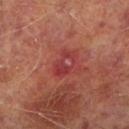Assessment:
Captured during whole-body skin photography for melanoma surveillance; the lesion was not biopsied.
Acquisition and patient details:
A male subject in their mid-60s. From the left lower leg. The total-body-photography lesion software estimated a shape-asymmetry score of about 0.25 (0 = symmetric). It also reported about 6 CIELAB-L* units darker than the surrounding skin. And it measured a border-irregularity index near 2/10, internal color variation of about 9.5 on a 0–10 scale, and radial color variation of about 3.5. The lesion's longest dimension is about 3 mm. A close-up tile cropped from a whole-body skin photograph, about 15 mm across. The tile uses cross-polarized illumination.